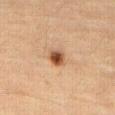Findings:
* patient — male, approximately 85 years of age or older
* image-analysis metrics — a lesion color around L≈45 a*≈20 b*≈32 in CIELAB, roughly 14 lightness units darker than nearby skin, and a normalized border contrast of about 11; a border-irregularity rating of about 2/10, a color-variation rating of about 4.5/10, and peripheral color asymmetry of about 1; a classifier nevus-likeness of about 100/100 and a detector confidence of about 100 out of 100 that the crop contains a lesion
* image source — ~15 mm tile from a whole-body skin photo
* illumination — cross-polarized
* body site — the abdomen
* size — ~2.5 mm (longest diameter)